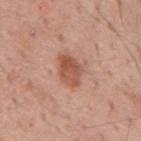Q: Was a biopsy performed?
A: imaged on a skin check; not biopsied
Q: Patient demographics?
A: male, roughly 60 years of age
Q: Lesion size?
A: ~4 mm (longest diameter)
Q: Illumination type?
A: white-light illumination
Q: Where on the body is the lesion?
A: the back
Q: What is the imaging modality?
A: 15 mm crop, total-body photography